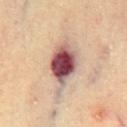workup: imaged on a skin check; not biopsied
patient: male, aged 63–67
site: the chest
image: ~15 mm tile from a whole-body skin photo